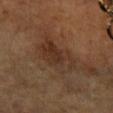Assessment: No biopsy was performed on this lesion — it was imaged during a full skin examination and was not determined to be concerning. Context: A female subject approximately 60 years of age. Located on the right forearm. The tile uses cross-polarized illumination. A close-up tile cropped from a whole-body skin photograph, about 15 mm across. Measured at roughly 6 mm in maximum diameter.